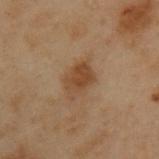Imaged during a routine full-body skin examination; the lesion was not biopsied and no histopathology is available. Imaged with cross-polarized lighting. Automated tile analysis of the lesion measured an area of roughly 7.5 mm² and a shape eccentricity near 0.75. The software also gave a lesion color around L≈36 a*≈15 b*≈28 in CIELAB, about 7 CIELAB-L* units darker than the surrounding skin, and a lesion-to-skin contrast of about 7.5 (normalized; higher = more distinct). The analysis additionally found a border-irregularity rating of about 2.5/10, internal color variation of about 3 on a 0–10 scale, and peripheral color asymmetry of about 1. The software also gave an automated nevus-likeness rating near 60 out of 100 and lesion-presence confidence of about 100/100. Cropped from a total-body skin-imaging series; the visible field is about 15 mm. On the upper back. A male patient, aged 53–57. Longest diameter approximately 4 mm.